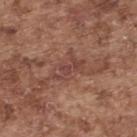workup: total-body-photography surveillance lesion; no biopsy | image source: total-body-photography crop, ~15 mm field of view | patient: male, about 75 years old | lesion diameter: ≈4 mm | body site: the upper back | tile lighting: white-light illumination.